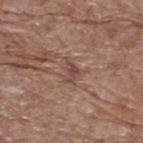Impression:
The lesion was photographed on a routine skin check and not biopsied; there is no pathology result.
Clinical summary:
The tile uses white-light illumination. A male subject, aged 68 to 72. Longest diameter approximately 3 mm. This image is a 15 mm lesion crop taken from a total-body photograph. On the upper back. The lesion-visualizer software estimated a footprint of about 3.5 mm² and an eccentricity of roughly 0.75. The analysis additionally found a mean CIELAB color near L≈46 a*≈20 b*≈23, about 8 CIELAB-L* units darker than the surrounding skin, and a normalized lesion–skin contrast near 6.5.